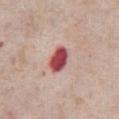Findings:
* tile lighting · white-light illumination
* size · about 3.5 mm
* patient · male, aged around 75
* imaging modality · ~15 mm tile from a whole-body skin photo
* location · the abdomen